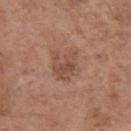workup — catalogued during a skin exam; not biopsied
lesion size — ≈4 mm
tile lighting — white-light
anatomic site — the left upper arm
imaging modality — 15 mm crop, total-body photography
patient — female, in their mid- to late 60s
TBP lesion metrics — an area of roughly 10 mm², a shape eccentricity near 0.55, and a shape-asymmetry score of about 0.35 (0 = symmetric); a lesion color around L≈50 a*≈21 b*≈27 in CIELAB, roughly 8 lightness units darker than nearby skin, and a normalized lesion–skin contrast near 5.5; a border-irregularity rating of about 4.5/10 and a within-lesion color-variation index near 3.5/10; an automated nevus-likeness rating near 5 out of 100 and lesion-presence confidence of about 100/100